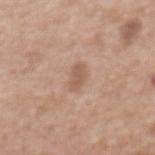Assessment:
Captured during whole-body skin photography for melanoma surveillance; the lesion was not biopsied.
Acquisition and patient details:
The lesion is located on the front of the torso. Automated image analysis of the tile measured an eccentricity of roughly 0.9. The software also gave a border-irregularity rating of about 3/10, internal color variation of about 0.5 on a 0–10 scale, and radial color variation of about 0. The software also gave a nevus-likeness score of about 5/100 and a lesion-detection confidence of about 100/100. A 15 mm crop from a total-body photograph taken for skin-cancer surveillance. Captured under white-light illumination. Measured at roughly 3 mm in maximum diameter. A female patient in their mid- to late 70s.